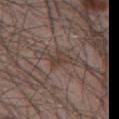Impression:
Captured during whole-body skin photography for melanoma surveillance; the lesion was not biopsied.
Clinical summary:
The recorded lesion diameter is about 3 mm. Automated image analysis of the tile measured a mean CIELAB color near L≈40 a*≈15 b*≈22 and a lesion-to-skin contrast of about 6 (normalized; higher = more distinct). And it measured a color-variation rating of about 2/10. And it measured a nevus-likeness score of about 0/100 and a lesion-detection confidence of about 80/100. A 15 mm crop from a total-body photograph taken for skin-cancer surveillance. The tile uses white-light illumination. The patient is a male roughly 40 years of age. On the chest.